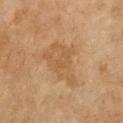Assessment:
Captured during whole-body skin photography for melanoma surveillance; the lesion was not biopsied.